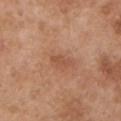Impression:
No biopsy was performed on this lesion — it was imaged during a full skin examination and was not determined to be concerning.
Clinical summary:
About 2.5 mm across. A close-up tile cropped from a whole-body skin photograph, about 15 mm across. The tile uses white-light illumination. The lesion is on the left upper arm. A male subject, in their mid-50s.